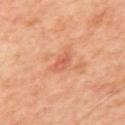Findings:
• follow-up: imaged on a skin check; not biopsied
• anatomic site: the back
• image: total-body-photography crop, ~15 mm field of view
• patient: male, aged 58 to 62
• diameter: about 3.5 mm